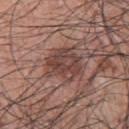This lesion was catalogued during total-body skin photography and was not selected for biopsy.
On the upper back.
An algorithmic analysis of the crop reported a mean CIELAB color near L≈43 a*≈20 b*≈22, a lesion–skin lightness drop of about 9, and a normalized border contrast of about 7.5. The software also gave border irregularity of about 2.5 on a 0–10 scale, a color-variation rating of about 4.5/10, and a peripheral color-asymmetry measure near 2. The software also gave a nevus-likeness score of about 5/100 and lesion-presence confidence of about 100/100.
The recorded lesion diameter is about 4.5 mm.
A male subject in their 70s.
Imaged with white-light lighting.
This image is a 15 mm lesion crop taken from a total-body photograph.